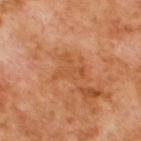This lesion was catalogued during total-body skin photography and was not selected for biopsy. Located on the upper back. A region of skin cropped from a whole-body photographic capture, roughly 15 mm wide. A male subject approximately 70 years of age.